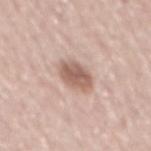notes: catalogued during a skin exam; not biopsied
size: ≈4 mm
imaging modality: ~15 mm crop, total-body skin-cancer survey
body site: the mid back
image-analysis metrics: a border-irregularity rating of about 1.5/10, a within-lesion color-variation index near 3.5/10, and a peripheral color-asymmetry measure near 1; an automated nevus-likeness rating near 85 out of 100 and a lesion-detection confidence of about 100/100
lighting: white-light
subject: male, aged around 60